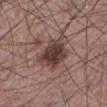The lesion was photographed on a routine skin check and not biopsied; there is no pathology result. This image is a 15 mm lesion crop taken from a total-body photograph. The lesion is on the left lower leg. Automated tile analysis of the lesion measured a lesion area of about 13 mm² and a shape eccentricity near 0.75. And it measured a lesion color around L≈39 a*≈17 b*≈19 in CIELAB, roughly 14 lightness units darker than nearby skin, and a normalized border contrast of about 11. The software also gave a border-irregularity index near 3/10, a within-lesion color-variation index near 5/10, and a peripheral color-asymmetry measure near 1.5. Imaged with white-light lighting. A male patient, about 60 years old.